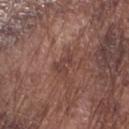notes=catalogued during a skin exam; not biopsied
subject=male, aged 73 to 77
location=the right forearm
image source=total-body-photography crop, ~15 mm field of view